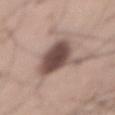Clinical impression:
Imaged during a routine full-body skin examination; the lesion was not biopsied and no histopathology is available.
Background:
A lesion tile, about 15 mm wide, cut from a 3D total-body photograph. The lesion's longest dimension is about 7 mm. On the abdomen. A male patient, approximately 65 years of age. Captured under white-light illumination.